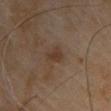Impression: The lesion was tiled from a total-body skin photograph and was not biopsied. Context: Longest diameter approximately 3 mm. From the right upper arm. The patient is a male in their mid- to late 60s. This is a cross-polarized tile. A 15 mm close-up extracted from a 3D total-body photography capture.